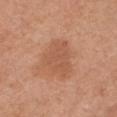{"biopsy_status": "not biopsied; imaged during a skin examination", "patient": {"sex": "female", "age_approx": 55}, "site": "chest", "image": {"source": "total-body photography crop", "field_of_view_mm": 15}}